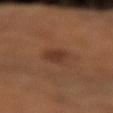The lesion's longest dimension is about 3 mm.
This is a cross-polarized tile.
A 15 mm close-up tile from a total-body photography series done for melanoma screening.
The patient is a female roughly 55 years of age.
On the right forearm.